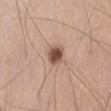This lesion was catalogued during total-body skin photography and was not selected for biopsy.
The subject is a male in their mid- to late 20s.
Measured at roughly 2.5 mm in maximum diameter.
This is a white-light tile.
Located on the abdomen.
A region of skin cropped from a whole-body photographic capture, roughly 15 mm wide.